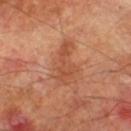Q: Was this lesion biopsied?
A: imaged on a skin check; not biopsied
Q: Patient demographics?
A: male, aged approximately 70
Q: What is the imaging modality?
A: total-body-photography crop, ~15 mm field of view
Q: Lesion location?
A: the right lower leg
Q: What is the lesion's diameter?
A: about 5.5 mm
Q: Illumination type?
A: cross-polarized illumination